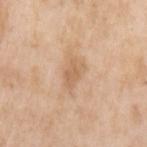Clinical impression:
Captured during whole-body skin photography for melanoma surveillance; the lesion was not biopsied.
Image and clinical context:
Imaged with white-light lighting. A female subject, roughly 75 years of age. Cropped from a total-body skin-imaging series; the visible field is about 15 mm. About 3 mm across. From the arm.